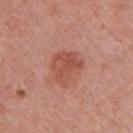biopsy_status: not biopsied; imaged during a skin examination
site: right upper arm
patient:
  sex: female
  age_approx: 40
image:
  source: total-body photography crop
  field_of_view_mm: 15